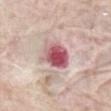biopsy status: no biopsy performed (imaged during a skin exam)
size: ~4 mm (longest diameter)
illumination: white-light
imaging modality: total-body-photography crop, ~15 mm field of view
patient: male, roughly 85 years of age or older
site: the front of the torso